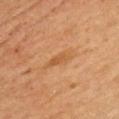follow-up: no biopsy performed (imaged during a skin exam) | site: the chest | patient: male, in their mid- to late 40s | image source: 15 mm crop, total-body photography.